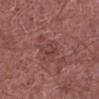A region of skin cropped from a whole-body photographic capture, roughly 15 mm wide. Measured at roughly 3 mm in maximum diameter. A male subject, aged 63–67. The tile uses white-light illumination. Located on the left lower leg.